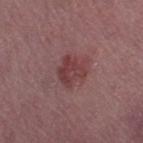<record>
<patient>
  <sex>female</sex>
  <age_approx>55</age_approx>
</patient>
<lesion_size>
  <long_diameter_mm_approx>3.5</long_diameter_mm_approx>
</lesion_size>
<automated_metrics>
  <area_mm2_approx>7.5</area_mm2_approx>
  <eccentricity>0.2</eccentricity>
  <shape_asymmetry>0.35</shape_asymmetry>
</automated_metrics>
<image>
  <source>total-body photography crop</source>
  <field_of_view_mm>15</field_of_view_mm>
</image>
<lighting>white-light</lighting>
<site>left thigh</site>
</record>